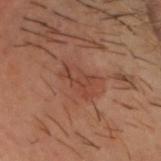Captured during whole-body skin photography for melanoma surveillance; the lesion was not biopsied. A roughly 15 mm field-of-view crop from a total-body skin photograph. Approximately 4.5 mm at its widest. Automated image analysis of the tile measured a footprint of about 8.5 mm², an outline eccentricity of about 0.85 (0 = round, 1 = elongated), and two-axis asymmetry of about 0.35. The software also gave a border-irregularity rating of about 4.5/10, a color-variation rating of about 3/10, and radial color variation of about 1. On the head or neck. A male patient approximately 50 years of age.